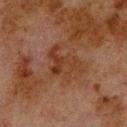Case summary:
* size — ≈5 mm
* image source — 15 mm crop, total-body photography
* subject — male, aged around 80
* tile lighting — cross-polarized
* anatomic site — the back